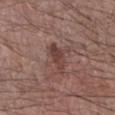{
  "biopsy_status": "not biopsied; imaged during a skin examination",
  "site": "right forearm",
  "image": {
    "source": "total-body photography crop",
    "field_of_view_mm": 15
  },
  "lesion_size": {
    "long_diameter_mm_approx": 4.0
  },
  "patient": {
    "sex": "male",
    "age_approx": 70
  }
}